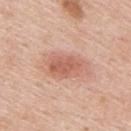Case summary:
- acquisition · 15 mm crop, total-body photography
- patient · male, aged 38 to 42
- size · about 5 mm
- body site · the arm